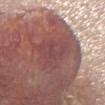Clinical impression:
The lesion was tiled from a total-body skin photograph and was not biopsied.
Clinical summary:
Measured at roughly 6 mm in maximum diameter. A close-up tile cropped from a whole-body skin photograph, about 15 mm across. Located on the chest. Captured under white-light illumination. A female subject aged approximately 55. An algorithmic analysis of the crop reported an eccentricity of roughly 0.6 and two-axis asymmetry of about 0.3. The software also gave an average lesion color of about L≈46 a*≈24 b*≈20 (CIELAB) and a normalized border contrast of about 5.5. The software also gave a border-irregularity rating of about 4/10 and radial color variation of about 1. And it measured an automated nevus-likeness rating near 0 out of 100.